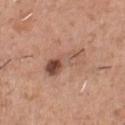Notes:
– notes — total-body-photography surveillance lesion; no biopsy
– subject — male, in their mid- to late 40s
– site — the chest
– image-analysis metrics — an area of roughly 9 mm², a shape eccentricity near 0.95, and a symmetry-axis asymmetry near 0.3; a border-irregularity index near 4.5/10, a within-lesion color-variation index near 10/10, and a peripheral color-asymmetry measure near 4.5
– acquisition — 15 mm crop, total-body photography
– size — ~5.5 mm (longest diameter)
– tile lighting — white-light